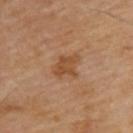Assessment:
The lesion was photographed on a routine skin check and not biopsied; there is no pathology result.
Background:
A 15 mm close-up extracted from a 3D total-body photography capture. Automated tile analysis of the lesion measured an area of roughly 6.5 mm², an outline eccentricity of about 0.7 (0 = round, 1 = elongated), and a shape-asymmetry score of about 0.3 (0 = symmetric). And it measured an average lesion color of about L≈47 a*≈21 b*≈34 (CIELAB), roughly 8 lightness units darker than nearby skin, and a normalized border contrast of about 7. Captured under cross-polarized illumination. Measured at roughly 3.5 mm in maximum diameter. The lesion is located on the back. The patient is a male aged around 70.